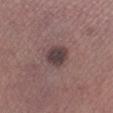workup: total-body-photography surveillance lesion; no biopsy | automated lesion analysis: border irregularity of about 1.5 on a 0–10 scale | lesion size: ~3 mm (longest diameter) | image: total-body-photography crop, ~15 mm field of view | patient: female, aged 38–42 | illumination: white-light illumination | anatomic site: the right lower leg.